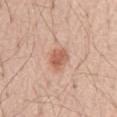biopsy_status: not biopsied; imaged during a skin examination
site: abdomen
image:
  source: total-body photography crop
  field_of_view_mm: 15
automated_metrics:
  eccentricity: 0.55
  shape_asymmetry: 0.2
  cielab_L: 60
  cielab_a: 24
  cielab_b: 31
  vs_skin_darker_L: 11.0
  vs_skin_contrast_norm: 7.5
  nevus_likeness_0_100: 95
patient:
  sex: male
  age_approx: 70
lesion_size:
  long_diameter_mm_approx: 3.0